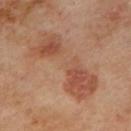Part of a total-body skin-imaging series; this lesion was reviewed on a skin check and was not flagged for biopsy.
The lesion-visualizer software estimated a footprint of about 26 mm² and two-axis asymmetry of about 0.35. It also reported a mean CIELAB color near L≈49 a*≈23 b*≈31 and a normalized border contrast of about 6.
A 15 mm close-up extracted from a 3D total-body photography capture.
The lesion is located on the mid back.
A male patient, roughly 70 years of age.
Imaged with cross-polarized lighting.